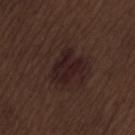Impression: No biopsy was performed on this lesion — it was imaged during a full skin examination and was not determined to be concerning. Image and clinical context: The total-body-photography lesion software estimated a footprint of about 9 mm², a shape eccentricity near 0.5, and a symmetry-axis asymmetry near 0.35. And it measured a lesion-detection confidence of about 100/100. Cropped from a whole-body photographic skin survey; the tile spans about 15 mm. Captured under white-light illumination. From the lower back. The lesion's longest dimension is about 4 mm. The patient is a male aged around 70.